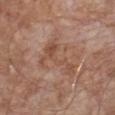{
  "biopsy_status": "not biopsied; imaged during a skin examination",
  "site": "chest",
  "patient": {
    "sex": "male",
    "age_approx": 65
  },
  "image": {
    "source": "total-body photography crop",
    "field_of_view_mm": 15
  },
  "lighting": "white-light",
  "lesion_size": {
    "long_diameter_mm_approx": 5.5
  },
  "automated_metrics": {
    "vs_skin_darker_L": 6.0,
    "border_irregularity_0_10": 8.5,
    "peripheral_color_asymmetry": 1.5,
    "nevus_likeness_0_100": 0,
    "lesion_detection_confidence_0_100": 100
  }
}